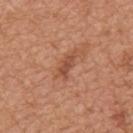Part of a total-body skin-imaging series; this lesion was reviewed on a skin check and was not flagged for biopsy.
Cropped from a total-body skin-imaging series; the visible field is about 15 mm.
A male subject, aged 63–67.
On the mid back.